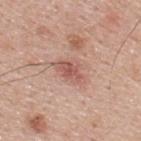notes: total-body-photography surveillance lesion; no biopsy
image: ~15 mm tile from a whole-body skin photo
lighting: white-light
lesion diameter: ≈4 mm
subject: male, approximately 40 years of age
site: the upper back